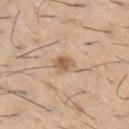The lesion was photographed on a routine skin check and not biopsied; there is no pathology result. A 15 mm close-up tile from a total-body photography series done for melanoma screening. From the front of the torso. An algorithmic analysis of the crop reported a mean CIELAB color near L≈59 a*≈18 b*≈33, roughly 11 lightness units darker than nearby skin, and a normalized border contrast of about 7.5. It also reported a border-irregularity index near 3/10, a within-lesion color-variation index near 3/10, and a peripheral color-asymmetry measure near 1. The subject is a male in their 60s.